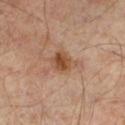Assessment: No biopsy was performed on this lesion — it was imaged during a full skin examination and was not determined to be concerning. Clinical summary: The subject is a male aged 48–52. This is a cross-polarized tile. A 15 mm crop from a total-body photograph taken for skin-cancer surveillance. From the left lower leg. Measured at roughly 3 mm in maximum diameter.